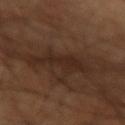| feature | finding |
|---|---|
| workup | no biopsy performed (imaged during a skin exam) |
| tile lighting | cross-polarized illumination |
| body site | the left forearm |
| imaging modality | ~15 mm crop, total-body skin-cancer survey |
| patient | male, about 60 years old |
| automated metrics | an automated nevus-likeness rating near 5 out of 100 and a detector confidence of about 85 out of 100 that the crop contains a lesion |